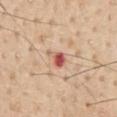Captured during whole-body skin photography for melanoma surveillance; the lesion was not biopsied. A male patient in their mid- to late 70s. A roughly 15 mm field-of-view crop from a total-body skin photograph. The lesion is on the abdomen.